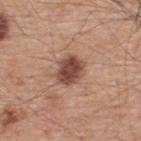notes=total-body-photography surveillance lesion; no biopsy | subject=male, approximately 55 years of age | diameter=~4 mm (longest diameter) | anatomic site=the upper back | image=15 mm crop, total-body photography.